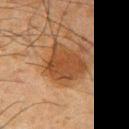Q: Was this lesion biopsied?
A: imaged on a skin check; not biopsied
Q: What kind of image is this?
A: 15 mm crop, total-body photography
Q: Lesion location?
A: the arm
Q: How large is the lesion?
A: about 4.5 mm
Q: What lighting was used for the tile?
A: cross-polarized
Q: What did automated image analysis measure?
A: an area of roughly 15 mm² and a shape eccentricity near 0.4; a mean CIELAB color near L≈36 a*≈19 b*≈30, roughly 8 lightness units darker than nearby skin, and a normalized lesion–skin contrast near 7.5; a border-irregularity index near 3/10; a classifier nevus-likeness of about 100/100 and a detector confidence of about 100 out of 100 that the crop contains a lesion
Q: Who is the patient?
A: male, aged 33 to 37